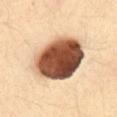{"biopsy_status": "not biopsied; imaged during a skin examination", "site": "abdomen", "image": {"source": "total-body photography crop", "field_of_view_mm": 15}, "patient": {"sex": "female", "age_approx": 35}}